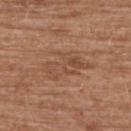No biopsy was performed on this lesion — it was imaged during a full skin examination and was not determined to be concerning.
A male subject, aged 73 to 77.
Located on the upper back.
Cropped from a whole-body photographic skin survey; the tile spans about 15 mm.
Longest diameter approximately 5.5 mm.
Captured under white-light illumination.